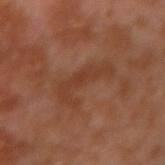Assessment: The lesion was tiled from a total-body skin photograph and was not biopsied. Image and clinical context: The lesion is on the left arm. A male subject, aged around 30. The tile uses cross-polarized illumination. The lesion's longest dimension is about 5.5 mm. Cropped from a total-body skin-imaging series; the visible field is about 15 mm.